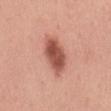Background:
The lesion is located on the mid back. Automated image analysis of the tile measured a lesion area of about 12 mm², an outline eccentricity of about 0.85 (0 = round, 1 = elongated), and a shape-asymmetry score of about 0.2 (0 = symmetric). And it measured a border-irregularity rating of about 2.5/10, a within-lesion color-variation index near 4/10, and radial color variation of about 1.5. Imaged with white-light lighting. A female patient aged around 55. A region of skin cropped from a whole-body photographic capture, roughly 15 mm wide. The lesion's longest dimension is about 5.5 mm.